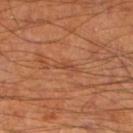| key | value |
|---|---|
| biopsy status | no biopsy performed (imaged during a skin exam) |
| subject | male, approximately 65 years of age |
| size | ~2.5 mm (longest diameter) |
| illumination | cross-polarized |
| image source | ~15 mm tile from a whole-body skin photo |
| anatomic site | the left lower leg |